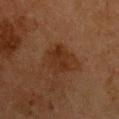{
  "biopsy_status": "not biopsied; imaged during a skin examination",
  "site": "front of the torso",
  "lighting": "cross-polarized",
  "patient": {
    "sex": "female",
    "age_approx": 50
  },
  "image": {
    "source": "total-body photography crop",
    "field_of_view_mm": 15
  }
}